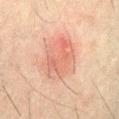Impression:
Imaged during a routine full-body skin examination; the lesion was not biopsied and no histopathology is available.
Context:
The lesion is located on the abdomen. An algorithmic analysis of the crop reported a footprint of about 20 mm², an eccentricity of roughly 0.55, and a shape-asymmetry score of about 0.15 (0 = symmetric). The software also gave a lesion color around L≈51 a*≈20 b*≈25 in CIELAB, a lesion–skin lightness drop of about 7, and a lesion-to-skin contrast of about 5.5 (normalized; higher = more distinct). The analysis additionally found a classifier nevus-likeness of about 20/100 and lesion-presence confidence of about 100/100. A region of skin cropped from a whole-body photographic capture, roughly 15 mm wide. About 5.5 mm across. A male subject, aged approximately 50. Captured under cross-polarized illumination.